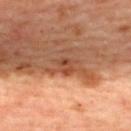{"biopsy_status": "not biopsied; imaged during a skin examination", "site": "upper back", "lesion_size": {"long_diameter_mm_approx": 2.5}, "patient": {"sex": "female", "age_approx": 45}, "image": {"source": "total-body photography crop", "field_of_view_mm": 15}, "lighting": "cross-polarized"}